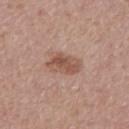follow-up = no biopsy performed (imaged during a skin exam) | lesion size = ~4 mm (longest diameter) | acquisition = 15 mm crop, total-body photography | tile lighting = white-light | site = the mid back | subject = male, approximately 60 years of age.